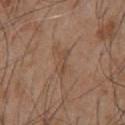biopsy status — no biopsy performed (imaged during a skin exam); body site — the chest; imaging modality — ~15 mm crop, total-body skin-cancer survey; illumination — white-light illumination; lesion size — ~3 mm (longest diameter); subject — male, aged 53–57.